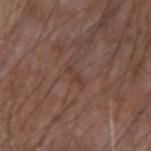Findings:
* notes · imaged on a skin check; not biopsied
* lesion diameter · ~2.5 mm (longest diameter)
* illumination · white-light
* patient · male, aged approximately 75
* site · the left forearm
* TBP lesion metrics · an eccentricity of roughly 0.9; a nevus-likeness score of about 0/100 and a detector confidence of about 95 out of 100 that the crop contains a lesion
* image · 15 mm crop, total-body photography